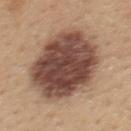Imaged with white-light lighting. Cropped from a whole-body photographic skin survey; the tile spans about 15 mm. Located on the upper back. A female subject aged 43–47.